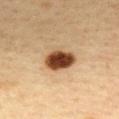{
  "biopsy_status": "not biopsied; imaged during a skin examination",
  "lighting": "cross-polarized",
  "image": {
    "source": "total-body photography crop",
    "field_of_view_mm": 15
  },
  "patient": {
    "sex": "female",
    "age_approx": 45
  },
  "lesion_size": {
    "long_diameter_mm_approx": 4.0
  },
  "site": "upper back"
}